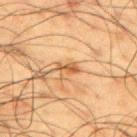The lesion was tiled from a total-body skin photograph and was not biopsied. Measured at roughly 2.5 mm in maximum diameter. A 15 mm crop from a total-body photograph taken for skin-cancer surveillance. The lesion is located on the back. The patient is a male in their 60s. Captured under cross-polarized illumination.